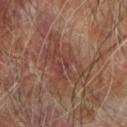Q: Was a biopsy performed?
A: imaged on a skin check; not biopsied
Q: What is the anatomic site?
A: the right forearm
Q: Who is the patient?
A: male, in their 70s
Q: What kind of image is this?
A: 15 mm crop, total-body photography